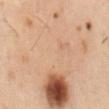Impression: Recorded during total-body skin imaging; not selected for excision or biopsy. Image and clinical context: The recorded lesion diameter is about 15.5 mm. The lesion is on the mid back. Imaged with cross-polarized lighting. A male patient in their mid- to late 50s. A 15 mm crop from a total-body photograph taken for skin-cancer surveillance.